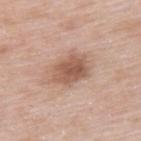biopsy_status: not biopsied; imaged during a skin examination
lighting: white-light
image:
  source: total-body photography crop
  field_of_view_mm: 15
site: upper back
lesion_size:
  long_diameter_mm_approx: 4.5
patient:
  sex: male
  age_approx: 55